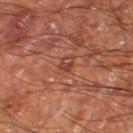notes: catalogued during a skin exam; not biopsied | subject: male, aged 58 to 62 | diameter: ~1.5 mm (longest diameter) | body site: the right thigh | acquisition: 15 mm crop, total-body photography.